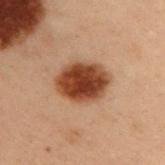| feature | finding |
|---|---|
| notes | total-body-photography surveillance lesion; no biopsy |
| automated lesion analysis | roughly 16 lightness units darker than nearby skin and a lesion-to-skin contrast of about 13 (normalized; higher = more distinct); a classifier nevus-likeness of about 100/100 and lesion-presence confidence of about 100/100 |
| anatomic site | the right upper arm |
| lesion diameter | ≈5 mm |
| imaging modality | 15 mm crop, total-body photography |
| patient | male, aged 53 to 57 |